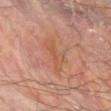Assessment:
The lesion was photographed on a routine skin check and not biopsied; there is no pathology result.
Context:
This image is a 15 mm lesion crop taken from a total-body photograph. The lesion is on the arm. The lesion-visualizer software estimated an eccentricity of roughly 0.85 and a shape-asymmetry score of about 0.5 (0 = symmetric). The analysis additionally found a lesion color around L≈46 a*≈21 b*≈29 in CIELAB, a lesion–skin lightness drop of about 5, and a normalized border contrast of about 5. And it measured lesion-presence confidence of about 100/100. A male subject in their mid-70s. Captured under cross-polarized illumination. Approximately 4.5 mm at its widest.